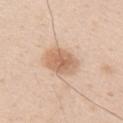Q: Was a biopsy performed?
A: catalogued during a skin exam; not biopsied
Q: Where on the body is the lesion?
A: the right upper arm
Q: Patient demographics?
A: male, roughly 30 years of age
Q: What kind of image is this?
A: ~15 mm crop, total-body skin-cancer survey